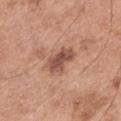Recorded during total-body skin imaging; not selected for excision or biopsy. Imaged with white-light lighting. Automated tile analysis of the lesion measured a lesion area of about 8 mm². The software also gave a classifier nevus-likeness of about 40/100 and a detector confidence of about 100 out of 100 that the crop contains a lesion. Located on the left thigh. The subject is a male roughly 65 years of age. The recorded lesion diameter is about 3.5 mm. A 15 mm crop from a total-body photograph taken for skin-cancer surveillance.